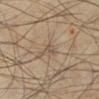notes: total-body-photography surveillance lesion; no biopsy | subject: male, in their mid-50s | lighting: cross-polarized illumination | body site: the left lower leg | image source: 15 mm crop, total-body photography | lesion diameter: ≈2.5 mm | automated lesion analysis: a shape eccentricity near 0.8 and two-axis asymmetry of about 0.45; lesion-presence confidence of about 10/100.